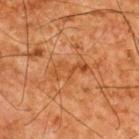biopsy_status: not biopsied; imaged during a skin examination